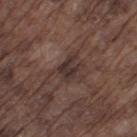| key | value |
|---|---|
| notes | no biopsy performed (imaged during a skin exam) |
| subject | male, in their mid- to late 70s |
| site | the left thigh |
| lighting | white-light |
| diameter | ~3 mm (longest diameter) |
| acquisition | ~15 mm crop, total-body skin-cancer survey |